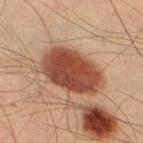Captured during whole-body skin photography for melanoma surveillance; the lesion was not biopsied. The patient is a male aged 38–42. A region of skin cropped from a whole-body photographic capture, roughly 15 mm wide. Automated image analysis of the tile measured a footprint of about 26 mm², an eccentricity of roughly 0.8, and a symmetry-axis asymmetry near 0.1. It also reported a border-irregularity rating of about 1.5/10, a color-variation rating of about 4.5/10, and a peripheral color-asymmetry measure near 1.5. This is a cross-polarized tile. From the left thigh. The lesion's longest dimension is about 7 mm.